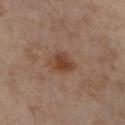{"biopsy_status": "not biopsied; imaged during a skin examination", "patient": {"sex": "female", "age_approx": 55}, "image": {"source": "total-body photography crop", "field_of_view_mm": 15}, "site": "right lower leg"}